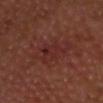A close-up tile cropped from a whole-body skin photograph, about 15 mm across. The total-body-photography lesion software estimated a within-lesion color-variation index near 3.5/10 and a peripheral color-asymmetry measure near 1. The analysis additionally found a nevus-likeness score of about 0/100 and lesion-presence confidence of about 100/100. A male subject in their mid-60s. The tile uses cross-polarized illumination. The lesion is on the head or neck.